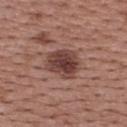workup: catalogued during a skin exam; not biopsied | anatomic site: the upper back | subject: female, in their 50s | diameter: ≈4 mm | illumination: white-light illumination | image: total-body-photography crop, ~15 mm field of view.